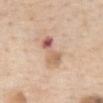Recorded during total-body skin imaging; not selected for excision or biopsy. A female subject aged 63–67. On the front of the torso. Cropped from a whole-body photographic skin survey; the tile spans about 15 mm. Automated image analysis of the tile measured a lesion area of about 7.5 mm² and a shape-asymmetry score of about 0.25 (0 = symmetric). And it measured a lesion color around L≈62 a*≈21 b*≈29 in CIELAB. It also reported a border-irregularity rating of about 4/10, internal color variation of about 10 on a 0–10 scale, and peripheral color asymmetry of about 4. The software also gave a nevus-likeness score of about 0/100 and a detector confidence of about 100 out of 100 that the crop contains a lesion. Captured under white-light illumination.